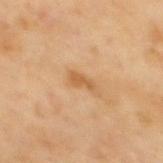follow-up: catalogued during a skin exam; not biopsied | site: the mid back | lighting: cross-polarized illumination | lesion size: ~2.5 mm (longest diameter) | imaging modality: 15 mm crop, total-body photography | patient: male, about 70 years old.